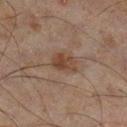Clinical impression:
This lesion was catalogued during total-body skin photography and was not selected for biopsy.
Background:
Cropped from a whole-body photographic skin survey; the tile spans about 15 mm. The lesion's longest dimension is about 3 mm. Imaged with cross-polarized lighting. The lesion is on the right lower leg. A male patient, in their mid- to late 40s.